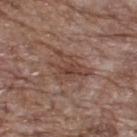biopsy status=imaged on a skin check; not biopsied | patient=male, approximately 70 years of age | acquisition=~15 mm crop, total-body skin-cancer survey | site=the upper back.